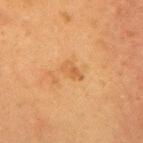This lesion was catalogued during total-body skin photography and was not selected for biopsy. A lesion tile, about 15 mm wide, cut from a 3D total-body photograph. The recorded lesion diameter is about 2.5 mm. From the mid back. The lesion-visualizer software estimated an eccentricity of roughly 0.85 and a symmetry-axis asymmetry near 0.35. And it measured a nevus-likeness score of about 0/100. The patient is a female aged approximately 50. Captured under cross-polarized illumination.